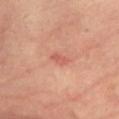Findings:
- workup · catalogued during a skin exam; not biopsied
- patient · female, aged approximately 40
- location · the chest
- tile lighting · cross-polarized
- size · about 2.5 mm
- imaging modality · 15 mm crop, total-body photography